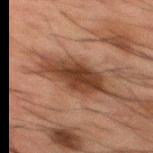Clinical impression:
The lesion was photographed on a routine skin check and not biopsied; there is no pathology result.
Acquisition and patient details:
Imaged with cross-polarized lighting. Cropped from a whole-body photographic skin survey; the tile spans about 15 mm. The patient is a male about 50 years old. From the mid back. Approximately 6 mm at its widest.